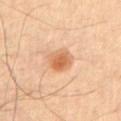This lesion was catalogued during total-body skin photography and was not selected for biopsy.
The subject is a male roughly 55 years of age.
Located on the chest.
A close-up tile cropped from a whole-body skin photograph, about 15 mm across.